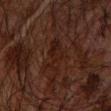The lesion was photographed on a routine skin check and not biopsied; there is no pathology result.
A male subject aged approximately 65.
The recorded lesion diameter is about 4 mm.
This is a cross-polarized tile.
From the left forearm.
A 15 mm crop from a total-body photograph taken for skin-cancer surveillance.